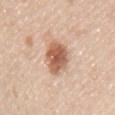biopsy status: imaged on a skin check; not biopsied
image source: ~15 mm crop, total-body skin-cancer survey
lesion size: ~4.5 mm (longest diameter)
site: the chest
image-analysis metrics: a lesion color around L≈60 a*≈22 b*≈31 in CIELAB and a lesion-to-skin contrast of about 9.5 (normalized; higher = more distinct); border irregularity of about 2 on a 0–10 scale, internal color variation of about 5.5 on a 0–10 scale, and peripheral color asymmetry of about 2
subject: male, approximately 45 years of age
tile lighting: white-light